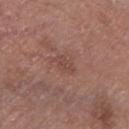follow-up = no biopsy performed (imaged during a skin exam); image source = ~15 mm tile from a whole-body skin photo; body site = the left lower leg; patient = male, aged approximately 80.